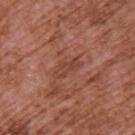Clinical impression:
Captured during whole-body skin photography for melanoma surveillance; the lesion was not biopsied.
Clinical summary:
This is a white-light tile. The patient is a male aged approximately 75. Located on the upper back. The total-body-photography lesion software estimated a border-irregularity index near 3.5/10 and peripheral color asymmetry of about 1. Cropped from a total-body skin-imaging series; the visible field is about 15 mm. The lesion's longest dimension is about 4 mm.